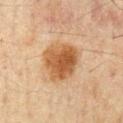Captured during whole-body skin photography for melanoma surveillance; the lesion was not biopsied. A 15 mm close-up extracted from a 3D total-body photography capture. Imaged with cross-polarized lighting. The patient is a male approximately 60 years of age.